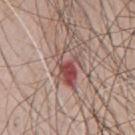Clinical impression: This lesion was catalogued during total-body skin photography and was not selected for biopsy. Acquisition and patient details: Located on the front of the torso. Captured under white-light illumination. A male subject, aged approximately 65. A roughly 15 mm field-of-view crop from a total-body skin photograph. The lesion's longest dimension is about 5 mm.